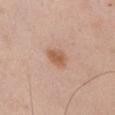Impression: Imaged during a routine full-body skin examination; the lesion was not biopsied and no histopathology is available. Image and clinical context: The patient is a male approximately 50 years of age. A region of skin cropped from a whole-body photographic capture, roughly 15 mm wide. The lesion is located on the left upper arm.